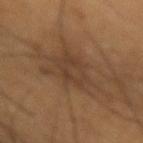Recorded during total-body skin imaging; not selected for excision or biopsy. The lesion-visualizer software estimated an outline eccentricity of about 0.65 (0 = round, 1 = elongated) and a symmetry-axis asymmetry near 0.5. The software also gave a mean CIELAB color near L≈35 a*≈14 b*≈26 and a lesion-to-skin contrast of about 5.5 (normalized; higher = more distinct). From the arm. Captured under cross-polarized illumination. About 7 mm across. A 15 mm close-up extracted from a 3D total-body photography capture. The patient is a male about 55 years old.